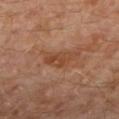Assessment:
This lesion was catalogued during total-body skin photography and was not selected for biopsy.
Acquisition and patient details:
The lesion is located on the left lower leg. A male patient roughly 30 years of age. Approximately 4 mm at its widest. Cropped from a whole-body photographic skin survey; the tile spans about 15 mm.